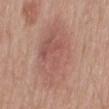<tbp_lesion>
<biopsy_status>not biopsied; imaged during a skin examination</biopsy_status>
<site>mid back</site>
<lesion_size>
  <long_diameter_mm_approx>9.0</long_diameter_mm_approx>
</lesion_size>
<image>
  <source>total-body photography crop</source>
  <field_of_view_mm>15</field_of_view_mm>
</image>
<lighting>white-light</lighting>
<automated_metrics>
  <cielab_L>56</cielab_L>
  <cielab_a>22</cielab_a>
  <cielab_b>25</cielab_b>
  <vs_skin_darker_L>7.0</vs_skin_darker_L>
  <nevus_likeness_0_100>0</nevus_likeness_0_100>
</automated_metrics>
<patient>
  <sex>male</sex>
  <age_approx>70</age_approx>
</patient>
</tbp_lesion>